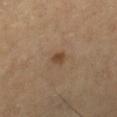biopsy status = catalogued during a skin exam; not biopsied | lighting = cross-polarized illumination | site = the left leg | image-analysis metrics = a lesion color around L≈44 a*≈18 b*≈32 in CIELAB, roughly 10 lightness units darker than nearby skin, and a normalized lesion–skin contrast near 8; a border-irregularity index near 2/10, a color-variation rating of about 1/10, and a peripheral color-asymmetry measure near 0.5; a classifier nevus-likeness of about 90/100 and lesion-presence confidence of about 100/100 | lesion diameter = about 2 mm | subject = female, roughly 60 years of age | image = ~15 mm tile from a whole-body skin photo.